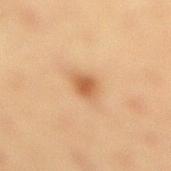Assessment:
Imaged during a routine full-body skin examination; the lesion was not biopsied and no histopathology is available.
Context:
Measured at roughly 2.5 mm in maximum diameter. A female subject, roughly 40 years of age. Cropped from a whole-body photographic skin survey; the tile spans about 15 mm. On the right lower leg. Automated image analysis of the tile measured a lesion area of about 4.5 mm², an eccentricity of roughly 0.55, and two-axis asymmetry of about 0.2. The analysis additionally found a mean CIELAB color near L≈51 a*≈20 b*≈35, about 10 CIELAB-L* units darker than the surrounding skin, and a normalized lesion–skin contrast near 7.5. The analysis additionally found a classifier nevus-likeness of about 90/100 and a detector confidence of about 100 out of 100 that the crop contains a lesion.